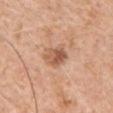Imaged during a routine full-body skin examination; the lesion was not biopsied and no histopathology is available.
Approximately 3 mm at its widest.
The lesion is located on the arm.
The tile uses white-light illumination.
A male subject aged approximately 60.
Automated tile analysis of the lesion measured an average lesion color of about L≈56 a*≈23 b*≈33 (CIELAB), about 12 CIELAB-L* units darker than the surrounding skin, and a normalized border contrast of about 8. The analysis additionally found border irregularity of about 2 on a 0–10 scale and a peripheral color-asymmetry measure near 2. And it measured a nevus-likeness score of about 15/100 and a lesion-detection confidence of about 100/100.
A lesion tile, about 15 mm wide, cut from a 3D total-body photograph.